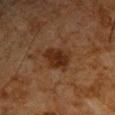The lesion was photographed on a routine skin check and not biopsied; there is no pathology result. From the front of the torso. A region of skin cropped from a whole-body photographic capture, roughly 15 mm wide. A male subject roughly 65 years of age. Longest diameter approximately 4.5 mm. This is a cross-polarized tile.